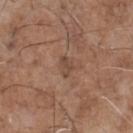| feature | finding |
|---|---|
| biopsy status | catalogued during a skin exam; not biopsied |
| image | 15 mm crop, total-body photography |
| patient | male, approximately 70 years of age |
| diameter | ≈2.5 mm |
| site | the chest |